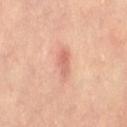The lesion was tiled from a total-body skin photograph and was not biopsied.
The lesion is on the lower back.
A female patient, in their mid- to late 30s.
Automated tile analysis of the lesion measured a shape eccentricity near 0.85 and two-axis asymmetry of about 0.25. The software also gave an automated nevus-likeness rating near 5 out of 100.
The lesion's longest dimension is about 3.5 mm.
A roughly 15 mm field-of-view crop from a total-body skin photograph.